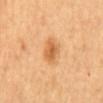| field | value |
|---|---|
| size | about 4 mm |
| image source | ~15 mm tile from a whole-body skin photo |
| patient | female, roughly 60 years of age |
| site | the mid back |
| automated metrics | an average lesion color of about L≈65 a*≈25 b*≈45 (CIELAB), a lesion–skin lightness drop of about 11, and a lesion-to-skin contrast of about 7 (normalized; higher = more distinct); a peripheral color-asymmetry measure near 1; an automated nevus-likeness rating near 90 out of 100 and a lesion-detection confidence of about 100/100 |
| illumination | cross-polarized |